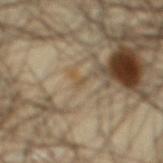<tbp_lesion>
  <biopsy_status>not biopsied; imaged during a skin examination</biopsy_status>
  <patient>
    <sex>male</sex>
    <age_approx>40</age_approx>
  </patient>
  <site>abdomen</site>
  <automated_metrics>
    <eccentricity>0.9</eccentricity>
    <shape_asymmetry>0.55</shape_asymmetry>
    <cielab_L>49</cielab_L>
    <cielab_a>11</cielab_a>
    <cielab_b>32</cielab_b>
    <vs_skin_darker_L>6.0</vs_skin_darker_L>
    <vs_skin_contrast_norm>5.0</vs_skin_contrast_norm>
    <nevus_likeness_0_100>0</nevus_likeness_0_100>
    <lesion_detection_confidence_0_100>5</lesion_detection_confidence_0_100>
  </automated_metrics>
  <lesion_size>
    <long_diameter_mm_approx>1.0</long_diameter_mm_approx>
  </lesion_size>
  <lighting>cross-polarized</lighting>
  <image>
    <source>total-body photography crop</source>
    <field_of_view_mm>15</field_of_view_mm>
  </image>
</tbp_lesion>